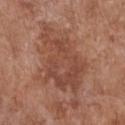No biopsy was performed on this lesion — it was imaged during a full skin examination and was not determined to be concerning. The lesion is on the chest. A 15 mm crop from a total-body photograph taken for skin-cancer surveillance. A female subject aged 73 to 77.